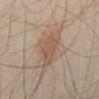Part of a total-body skin-imaging series; this lesion was reviewed on a skin check and was not flagged for biopsy. The lesion's longest dimension is about 4 mm. A male subject approximately 40 years of age. A 15 mm close-up tile from a total-body photography series done for melanoma screening. The tile uses cross-polarized illumination. The lesion is on the abdomen. An algorithmic analysis of the crop reported roughly 7 lightness units darker than nearby skin and a normalized border contrast of about 5.5. The analysis additionally found a border-irregularity index near 3/10 and a peripheral color-asymmetry measure near 1.